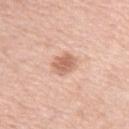Clinical impression:
Recorded during total-body skin imaging; not selected for excision or biopsy.
Image and clinical context:
Automated tile analysis of the lesion measured a nevus-likeness score of about 80/100 and a detector confidence of about 100 out of 100 that the crop contains a lesion. The lesion is located on the left upper arm. A male subject aged approximately 55. Approximately 3 mm at its widest. Cropped from a total-body skin-imaging series; the visible field is about 15 mm.